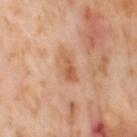Q: Was a biopsy performed?
A: imaged on a skin check; not biopsied
Q: How was this image acquired?
A: total-body-photography crop, ~15 mm field of view
Q: What did automated image analysis measure?
A: a border-irregularity index near 4/10, internal color variation of about 3 on a 0–10 scale, and radial color variation of about 1; a classifier nevus-likeness of about 0/100 and a detector confidence of about 100 out of 100 that the crop contains a lesion
Q: Illumination type?
A: cross-polarized illumination
Q: What is the lesion's diameter?
A: ≈3.5 mm
Q: What is the anatomic site?
A: the right thigh
Q: What are the patient's age and sex?
A: female, aged 53 to 57